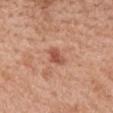Assessment:
This lesion was catalogued during total-body skin photography and was not selected for biopsy.
Acquisition and patient details:
Longest diameter approximately 2.5 mm. This image is a 15 mm lesion crop taken from a total-body photograph. A female patient, in their 40s. The tile uses white-light illumination. Located on the mid back. Automated tile analysis of the lesion measured an area of roughly 3.5 mm², an eccentricity of roughly 0.6, and a shape-asymmetry score of about 0.3 (0 = symmetric). The software also gave a lesion–skin lightness drop of about 11. The software also gave radial color variation of about 0.5. It also reported a nevus-likeness score of about 55/100.